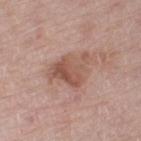Clinical impression: No biopsy was performed on this lesion — it was imaged during a full skin examination and was not determined to be concerning. Background: Located on the left thigh. A 15 mm crop from a total-body photograph taken for skin-cancer surveillance. The tile uses white-light illumination. The lesion's longest dimension is about 5 mm. A male patient, in their 70s.